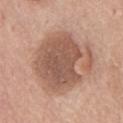follow-up: total-body-photography surveillance lesion; no biopsy | subject: male, roughly 75 years of age | body site: the chest | image source: total-body-photography crop, ~15 mm field of view.